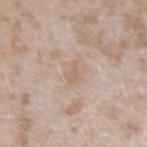Impression:
Recorded during total-body skin imaging; not selected for excision or biopsy.
Image and clinical context:
Cropped from a whole-body photographic skin survey; the tile spans about 15 mm. The patient is a female aged approximately 25. Measured at roughly 3 mm in maximum diameter. Imaged with white-light lighting. The lesion is on the right forearm.